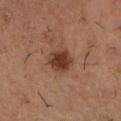Impression: Recorded during total-body skin imaging; not selected for excision or biopsy. Image and clinical context: On the left forearm. A 15 mm crop from a total-body photograph taken for skin-cancer surveillance. Longest diameter approximately 3.5 mm. The patient is a male approximately 55 years of age.